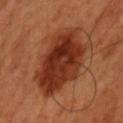The lesion was tiled from a total-body skin photograph and was not biopsied. From the left upper arm. Longest diameter approximately 8 mm. A male patient, roughly 50 years of age. This image is a 15 mm lesion crop taken from a total-body photograph. The lesion-visualizer software estimated a lesion color around L≈34 a*≈28 b*≈33 in CIELAB and a lesion-to-skin contrast of about 11 (normalized; higher = more distinct). It also reported a border-irregularity rating of about 2.5/10, a within-lesion color-variation index near 6/10, and a peripheral color-asymmetry measure near 2. The software also gave a classifier nevus-likeness of about 100/100 and a detector confidence of about 100 out of 100 that the crop contains a lesion.